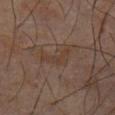notes = catalogued during a skin exam; not biopsied | patient = male, aged 58–62 | image source = ~15 mm tile from a whole-body skin photo | body site = the abdomen | automated lesion analysis = a mean CIELAB color near L≈35 a*≈14 b*≈24, about 4 CIELAB-L* units darker than the surrounding skin, and a normalized border contrast of about 5.5; a nevus-likeness score of about 0/100 and a detector confidence of about 100 out of 100 that the crop contains a lesion | lighting = cross-polarized illumination.